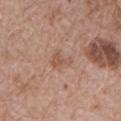Findings:
* notes: no biopsy performed (imaged during a skin exam)
* subject: male, in their mid- to late 60s
* image: 15 mm crop, total-body photography
* location: the chest
* lesion diameter: ≈2.5 mm
* tile lighting: white-light